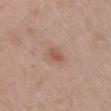Assessment:
The lesion was tiled from a total-body skin photograph and was not biopsied.
Clinical summary:
The lesion is on the left upper arm. The patient is a female aged 48–52. A 15 mm crop from a total-body photograph taken for skin-cancer surveillance.